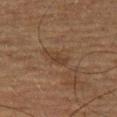Q: Is there a histopathology result?
A: imaged on a skin check; not biopsied
Q: What did automated image analysis measure?
A: a shape eccentricity near 0.85; a mean CIELAB color near L≈30 a*≈14 b*≈24, roughly 5 lightness units darker than nearby skin, and a normalized lesion–skin contrast near 5.5; a border-irregularity index near 3.5/10 and internal color variation of about 1 on a 0–10 scale; an automated nevus-likeness rating near 0 out of 100 and a detector confidence of about 100 out of 100 that the crop contains a lesion
Q: Patient demographics?
A: male, aged 73 to 77
Q: Lesion location?
A: the leg
Q: What is the imaging modality?
A: total-body-photography crop, ~15 mm field of view
Q: Illumination type?
A: cross-polarized illumination
Q: How large is the lesion?
A: ~2.5 mm (longest diameter)